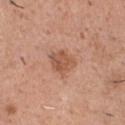Notes:
- notes · total-body-photography surveillance lesion; no biopsy
- lesion diameter · about 3 mm
- automated metrics · a mean CIELAB color near L≈55 a*≈23 b*≈32, about 10 CIELAB-L* units darker than the surrounding skin, and a normalized border contrast of about 7
- site · the chest
- patient · male, in their mid- to late 40s
- acquisition · ~15 mm tile from a whole-body skin photo
- illumination · white-light illumination